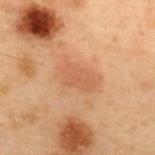Notes:
– location · the upper back
– image source · ~15 mm crop, total-body skin-cancer survey
– subject · male, in their mid-50s